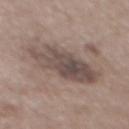• biopsy status · imaged on a skin check; not biopsied
• lesion diameter · about 8 mm
• subject · male, about 50 years old
• lighting · white-light
• image source · 15 mm crop, total-body photography
• automated lesion analysis · a border-irregularity index near 4/10, internal color variation of about 5.5 on a 0–10 scale, and peripheral color asymmetry of about 2
• site · the mid back